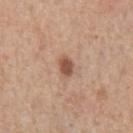workup: no biopsy performed (imaged during a skin exam).